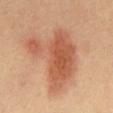{
  "biopsy_status": "not biopsied; imaged during a skin examination",
  "patient": {
    "sex": "male",
    "age_approx": 40
  },
  "image": {
    "source": "total-body photography crop",
    "field_of_view_mm": 15
  },
  "automated_metrics": {
    "area_mm2_approx": 31.0,
    "eccentricity": 0.6,
    "shape_asymmetry": 0.45,
    "vs_skin_darker_L": 11.0,
    "vs_skin_contrast_norm": 7.5
  },
  "lesion_size": {
    "long_diameter_mm_approx": 7.5
  },
  "site": "abdomen"
}